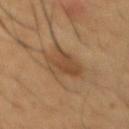Q: Was this lesion biopsied?
A: total-body-photography surveillance lesion; no biopsy
Q: How was the tile lit?
A: cross-polarized illumination
Q: Where on the body is the lesion?
A: the chest
Q: How large is the lesion?
A: about 4.5 mm
Q: Who is the patient?
A: male, in their mid-40s
Q: What kind of image is this?
A: total-body-photography crop, ~15 mm field of view